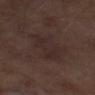Part of a total-body skin-imaging series; this lesion was reviewed on a skin check and was not flagged for biopsy. The subject is a male approximately 70 years of age. This is a cross-polarized tile. A region of skin cropped from a whole-body photographic capture, roughly 15 mm wide. The lesion is on the left thigh. Automated tile analysis of the lesion measured a footprint of about 13 mm², a shape eccentricity near 0.9, and a symmetry-axis asymmetry near 0.2. The software also gave a mean CIELAB color near L≈25 a*≈14 b*≈16, roughly 4 lightness units darker than nearby skin, and a lesion-to-skin contrast of about 5 (normalized; higher = more distinct). And it measured an automated nevus-likeness rating near 0 out of 100 and lesion-presence confidence of about 100/100.